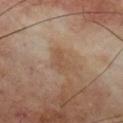Notes:
• workup: imaged on a skin check; not biopsied
• subject: male, approximately 70 years of age
• illumination: cross-polarized illumination
• image-analysis metrics: a lesion area of about 4 mm², an outline eccentricity of about 0.85 (0 = round, 1 = elongated), and a shape-asymmetry score of about 0.3 (0 = symmetric); an automated nevus-likeness rating near 0 out of 100 and a detector confidence of about 100 out of 100 that the crop contains a lesion
• lesion size: ≈3 mm
• anatomic site: the chest
• acquisition: total-body-photography crop, ~15 mm field of view